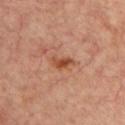Assessment: No biopsy was performed on this lesion — it was imaged during a full skin examination and was not determined to be concerning. Context: On the front of the torso. Cropped from a whole-body photographic skin survey; the tile spans about 15 mm. About 3 mm across. Imaged with cross-polarized lighting. A female subject approximately 45 years of age. The lesion-visualizer software estimated a lesion color around L≈47 a*≈25 b*≈33 in CIELAB and a lesion–skin lightness drop of about 10.